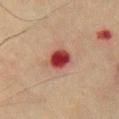Case summary:
* follow-up: catalogued during a skin exam; not biopsied
* lighting: cross-polarized illumination
* automated lesion analysis: a classifier nevus-likeness of about 0/100 and a detector confidence of about 100 out of 100 that the crop contains a lesion
* location: the chest
* patient: male, aged approximately 75
* image source: ~15 mm tile from a whole-body skin photo